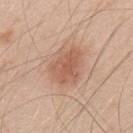Notes:
– biopsy status — catalogued during a skin exam; not biopsied
– site — the upper back
– image source — ~15 mm tile from a whole-body skin photo
– subject — male, about 50 years old